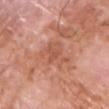Assessment:
No biopsy was performed on this lesion — it was imaged during a full skin examination and was not determined to be concerning.
Context:
About 5.5 mm across. From the front of the torso. This is a white-light tile. The subject is a male aged around 60. A lesion tile, about 15 mm wide, cut from a 3D total-body photograph.